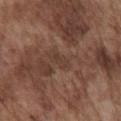Part of a total-body skin-imaging series; this lesion was reviewed on a skin check and was not flagged for biopsy.
Automated tile analysis of the lesion measured a lesion color around L≈38 a*≈18 b*≈24 in CIELAB, roughly 6 lightness units darker than nearby skin, and a normalized lesion–skin contrast near 5.
Longest diameter approximately 2.5 mm.
A region of skin cropped from a whole-body photographic capture, roughly 15 mm wide.
Imaged with white-light lighting.
From the chest.
A male subject, in their mid-70s.